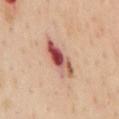Measured at roughly 6 mm in maximum diameter. Automated tile analysis of the lesion measured a lesion area of about 10 mm², an eccentricity of roughly 0.9, and two-axis asymmetry of about 0.2. And it measured a border-irregularity index near 3/10, a color-variation rating of about 10/10, and peripheral color asymmetry of about 7. A roughly 15 mm field-of-view crop from a total-body skin photograph. On the chest. A male subject, in their mid- to late 50s.